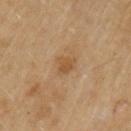| field | value |
|---|---|
| follow-up | catalogued during a skin exam; not biopsied |
| lighting | cross-polarized illumination |
| site | the left upper arm |
| acquisition | ~15 mm tile from a whole-body skin photo |
| patient | male, about 55 years old |
| size | about 2.5 mm |